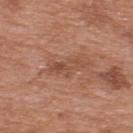Q: Was this lesion biopsied?
A: catalogued during a skin exam; not biopsied
Q: Patient demographics?
A: male, in their 70s
Q: What kind of image is this?
A: ~15 mm crop, total-body skin-cancer survey
Q: Illumination type?
A: white-light
Q: What is the anatomic site?
A: the upper back
Q: What is the lesion's diameter?
A: ~4.5 mm (longest diameter)